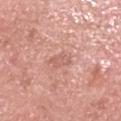The lesion was tiled from a total-body skin photograph and was not biopsied.